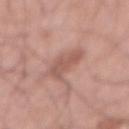Imaged during a routine full-body skin examination; the lesion was not biopsied and no histopathology is available. A male subject, aged 68–72. On the abdomen. This image is a 15 mm lesion crop taken from a total-body photograph.